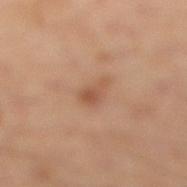Q: Is there a histopathology result?
A: imaged on a skin check; not biopsied
Q: Patient demographics?
A: male, approximately 50 years of age
Q: Where on the body is the lesion?
A: the left leg
Q: What is the lesion's diameter?
A: ~3 mm (longest diameter)
Q: How was this image acquired?
A: total-body-photography crop, ~15 mm field of view
Q: What lighting was used for the tile?
A: cross-polarized illumination